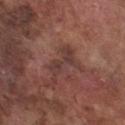notes — total-body-photography surveillance lesion; no biopsy | acquisition — ~15 mm tile from a whole-body skin photo | anatomic site — the chest | patient — male, in their mid- to late 70s | image-analysis metrics — an area of roughly 6.5 mm², an eccentricity of roughly 0.9, and a symmetry-axis asymmetry near 0.5 | size — ≈4.5 mm.